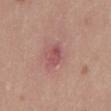{
  "biopsy_status": "not biopsied; imaged during a skin examination",
  "lesion_size": {
    "long_diameter_mm_approx": 3.0
  },
  "site": "mid back",
  "automated_metrics": {
    "nevus_likeness_0_100": 0
  },
  "patient": {
    "sex": "male",
    "age_approx": 25
  },
  "image": {
    "source": "total-body photography crop",
    "field_of_view_mm": 15
  }
}